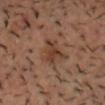workup: imaged on a skin check; not biopsied | location: the head or neck | lesion size: ≈4 mm | image source: ~15 mm crop, total-body skin-cancer survey | automated metrics: a footprint of about 6.5 mm² and a symmetry-axis asymmetry near 0.45; a lesion color around L≈37 a*≈19 b*≈27 in CIELAB and a normalized border contrast of about 7.5 | subject: male, aged 38–42.